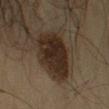Q: Is there a histopathology result?
A: no biopsy performed (imaged during a skin exam)
Q: Where on the body is the lesion?
A: the arm
Q: How large is the lesion?
A: about 6 mm
Q: What are the patient's age and sex?
A: male, in their mid-50s
Q: What is the imaging modality?
A: total-body-photography crop, ~15 mm field of view
Q: What lighting was used for the tile?
A: cross-polarized illumination